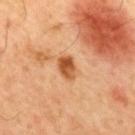follow-up = total-body-photography surveillance lesion; no biopsy
body site = the mid back
imaging modality = 15 mm crop, total-body photography
illumination = cross-polarized illumination
diameter = about 3 mm
TBP lesion metrics = a lesion color around L≈53 a*≈26 b*≈41 in CIELAB, a lesion–skin lightness drop of about 14, and a normalized lesion–skin contrast near 9.5; a nevus-likeness score of about 85/100
patient = male, aged 58–62